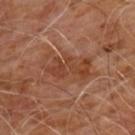Imaged during a routine full-body skin examination; the lesion was not biopsied and no histopathology is available.
On the chest.
A male patient roughly 60 years of age.
A 15 mm crop from a total-body photograph taken for skin-cancer surveillance.
Automated tile analysis of the lesion measured a footprint of about 16 mm², a shape eccentricity near 0.8, and two-axis asymmetry of about 0.25. The software also gave a detector confidence of about 100 out of 100 that the crop contains a lesion.